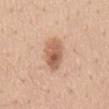Part of a total-body skin-imaging series; this lesion was reviewed on a skin check and was not flagged for biopsy. A male subject aged approximately 55. Automated tile analysis of the lesion measured a lesion area of about 10 mm², an eccentricity of roughly 0.85, and two-axis asymmetry of about 0.15. The software also gave a lesion color around L≈60 a*≈21 b*≈32 in CIELAB, roughly 13 lightness units darker than nearby skin, and a normalized border contrast of about 8. The software also gave a border-irregularity index near 2/10, a within-lesion color-variation index near 6.5/10, and peripheral color asymmetry of about 2. Located on the mid back. Longest diameter approximately 5 mm. A lesion tile, about 15 mm wide, cut from a 3D total-body photograph.